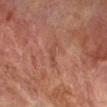Captured during whole-body skin photography for melanoma surveillance; the lesion was not biopsied.
A 15 mm close-up tile from a total-body photography series done for melanoma screening.
On the right forearm.
The recorded lesion diameter is about 3 mm.
The patient is a male aged around 70.